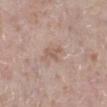Captured during whole-body skin photography for melanoma surveillance; the lesion was not biopsied.
The tile uses white-light illumination.
A 15 mm close-up extracted from a 3D total-body photography capture.
About 3 mm across.
A male subject roughly 50 years of age.
From the left lower leg.